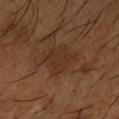Findings:
– biopsy status — total-body-photography surveillance lesion; no biopsy
– patient — male, in their mid- to late 60s
– anatomic site — the right forearm
– diameter — about 4 mm
– image — total-body-photography crop, ~15 mm field of view